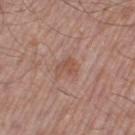notes: imaged on a skin check; not biopsied | TBP lesion metrics: a lesion color around L≈52 a*≈21 b*≈28 in CIELAB, about 7 CIELAB-L* units darker than the surrounding skin, and a lesion-to-skin contrast of about 5.5 (normalized; higher = more distinct); a classifier nevus-likeness of about 0/100 | image: 15 mm crop, total-body photography | lesion size: ≈2.5 mm | patient: male, approximately 55 years of age | site: the leg.